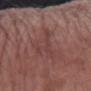<lesion>
  <biopsy_status>not biopsied; imaged during a skin examination</biopsy_status>
  <image>
    <source>total-body photography crop</source>
    <field_of_view_mm>15</field_of_view_mm>
  </image>
  <lesion_size>
    <long_diameter_mm_approx>6.0</long_diameter_mm_approx>
  </lesion_size>
  <site>right forearm</site>
  <lighting>white-light</lighting>
  <patient>
    <sex>female</sex>
    <age_approx>70</age_approx>
  </patient>
</lesion>